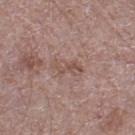{
  "biopsy_status": "not biopsied; imaged during a skin examination",
  "image": {
    "source": "total-body photography crop",
    "field_of_view_mm": 15
  },
  "site": "left thigh",
  "patient": {
    "sex": "male",
    "age_approx": 70
  }
}